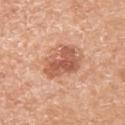biopsy status: catalogued during a skin exam; not biopsied
lighting: white-light illumination
image: total-body-photography crop, ~15 mm field of view
lesion size: ~4.5 mm (longest diameter)
site: the left upper arm
patient: female, approximately 75 years of age